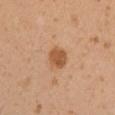Imaged during a routine full-body skin examination; the lesion was not biopsied and no histopathology is available. Cropped from a whole-body photographic skin survey; the tile spans about 15 mm. A female subject, approximately 30 years of age. Located on the right upper arm.